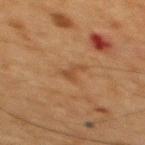<lesion>
<biopsy_status>not biopsied; imaged during a skin examination</biopsy_status>
<lesion_size>
  <long_diameter_mm_approx>3.0</long_diameter_mm_approx>
</lesion_size>
<patient>
  <sex>male</sex>
  <age_approx>65</age_approx>
</patient>
<site>upper back</site>
<lighting>cross-polarized</lighting>
<image>
  <source>total-body photography crop</source>
  <field_of_view_mm>15</field_of_view_mm>
</image>
</lesion>